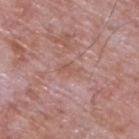Recorded during total-body skin imaging; not selected for excision or biopsy. Captured under white-light illumination. Longest diameter approximately 3 mm. An algorithmic analysis of the crop reported a footprint of about 3 mm² and a shape-asymmetry score of about 0.35 (0 = symmetric). And it measured a border-irregularity rating of about 4/10, a within-lesion color-variation index near 0/10, and a peripheral color-asymmetry measure near 0. The subject is a male aged approximately 65. From the upper back. A 15 mm close-up tile from a total-body photography series done for melanoma screening.